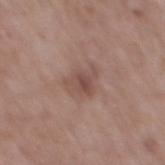{"image": {"source": "total-body photography crop", "field_of_view_mm": 15}, "site": "mid back", "lesion_size": {"long_diameter_mm_approx": 3.5}, "patient": {"sex": "male", "age_approx": 65}, "automated_metrics": {"area_mm2_approx": 7.0, "eccentricity": 0.7, "shape_asymmetry": 0.4, "cielab_L": 49, "cielab_a": 18, "cielab_b": 23, "vs_skin_darker_L": 8.0, "vs_skin_contrast_norm": 6.0, "lesion_detection_confidence_0_100": 100}}